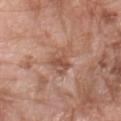Assessment: Recorded during total-body skin imaging; not selected for excision or biopsy. Image and clinical context: On the arm. Cropped from a whole-body photographic skin survey; the tile spans about 15 mm. A male patient, approximately 60 years of age.